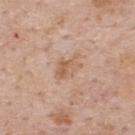This lesion was catalogued during total-body skin photography and was not selected for biopsy. The lesion is located on the back. A lesion tile, about 15 mm wide, cut from a 3D total-body photograph. The subject is a male approximately 60 years of age.